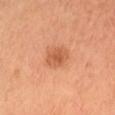Captured during whole-body skin photography for melanoma surveillance; the lesion was not biopsied.
A lesion tile, about 15 mm wide, cut from a 3D total-body photograph.
The lesion is on the head or neck.
Automated image analysis of the tile measured a color-variation rating of about 2.5/10 and a peripheral color-asymmetry measure near 0.5.
The tile uses cross-polarized illumination.
The recorded lesion diameter is about 3 mm.
A female patient, about 35 years old.